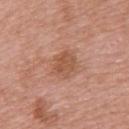follow-up — total-body-photography surveillance lesion; no biopsy
lesion size — about 3.5 mm
body site — the upper back
patient — female, aged 68 to 72
lighting — white-light
image source — 15 mm crop, total-body photography